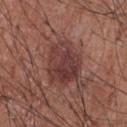Recorded during total-body skin imaging; not selected for excision or biopsy.
The recorded lesion diameter is about 5.5 mm.
From the front of the torso.
Automated image analysis of the tile measured an area of roughly 18 mm², a shape eccentricity near 0.65, and two-axis asymmetry of about 0.2. The software also gave border irregularity of about 2.5 on a 0–10 scale, internal color variation of about 5 on a 0–10 scale, and peripheral color asymmetry of about 2. And it measured an automated nevus-likeness rating near 40 out of 100 and lesion-presence confidence of about 100/100.
A lesion tile, about 15 mm wide, cut from a 3D total-body photograph.
The subject is a male aged 73–77.